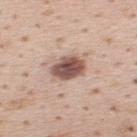  biopsy_status: not biopsied; imaged during a skin examination
  lesion_size:
    long_diameter_mm_approx: 4.0
  site: upper back
  lighting: white-light
  image:
    source: total-body photography crop
    field_of_view_mm: 15
  patient:
    sex: male
    age_approx: 35
  automated_metrics:
    cielab_L: 53
    cielab_a: 19
    cielab_b: 24
    vs_skin_darker_L: 18.0
    nevus_likeness_0_100: 70